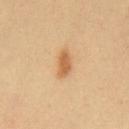Background:
Measured at roughly 3.5 mm in maximum diameter. A region of skin cropped from a whole-body photographic capture, roughly 15 mm wide. An algorithmic analysis of the crop reported a mean CIELAB color near L≈62 a*≈22 b*≈41. The software also gave a border-irregularity index near 2.5/10, a color-variation rating of about 2/10, and a peripheral color-asymmetry measure near 0.5. It also reported an automated nevus-likeness rating near 95 out of 100 and a lesion-detection confidence of about 100/100. A male subject, roughly 40 years of age. On the chest.